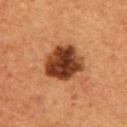Case summary:
• notes — imaged on a skin check; not biopsied
• patient — female, in their 40s
• image-analysis metrics — an automated nevus-likeness rating near 80 out of 100 and a detector confidence of about 100 out of 100 that the crop contains a lesion
• anatomic site — the upper back
• illumination — cross-polarized
• lesion size — about 5 mm
• acquisition — ~15 mm tile from a whole-body skin photo